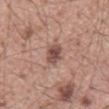The total-body-photography lesion software estimated a lesion color around L≈49 a*≈21 b*≈23 in CIELAB, roughly 12 lightness units darker than nearby skin, and a normalized border contrast of about 9. And it measured a border-irregularity index near 2.5/10, a within-lesion color-variation index near 3.5/10, and a peripheral color-asymmetry measure near 1. It also reported a classifier nevus-likeness of about 80/100 and a lesion-detection confidence of about 100/100. A male patient roughly 55 years of age. Captured under white-light illumination. The lesion is on the mid back. About 3.5 mm across. A region of skin cropped from a whole-body photographic capture, roughly 15 mm wide.